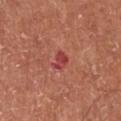The lesion's longest dimension is about 2.5 mm. Captured under white-light illumination. A male subject aged approximately 70. Located on the left lower leg. A close-up tile cropped from a whole-body skin photograph, about 15 mm across.